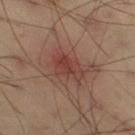Q: Illumination type?
A: cross-polarized illumination
Q: How was this image acquired?
A: ~15 mm crop, total-body skin-cancer survey
Q: What are the patient's age and sex?
A: male, roughly 40 years of age
Q: Lesion size?
A: ~4 mm (longest diameter)
Q: Lesion location?
A: the right thigh